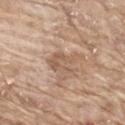<lesion>
<biopsy_status>not biopsied; imaged during a skin examination</biopsy_status>
<image>
  <source>total-body photography crop</source>
  <field_of_view_mm>15</field_of_view_mm>
</image>
<patient>
  <sex>male</sex>
  <age_approx>65</age_approx>
</patient>
<site>mid back</site>
</lesion>